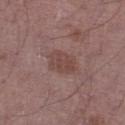workup=catalogued during a skin exam; not biopsied
anatomic site=the left lower leg
imaging modality=~15 mm crop, total-body skin-cancer survey
lesion diameter=about 4 mm
illumination=white-light
subject=male, about 50 years old
TBP lesion metrics=a lesion area of about 7.5 mm², a shape eccentricity near 0.75, and a symmetry-axis asymmetry near 0.2; a lesion–skin lightness drop of about 7 and a normalized lesion–skin contrast near 6; border irregularity of about 2 on a 0–10 scale, a within-lesion color-variation index near 2.5/10, and peripheral color asymmetry of about 1; an automated nevus-likeness rating near 0 out of 100 and lesion-presence confidence of about 100/100